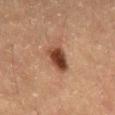follow-up = catalogued during a skin exam; not biopsied
lesion size = about 4 mm
subject = male, approximately 60 years of age
acquisition = total-body-photography crop, ~15 mm field of view
tile lighting = cross-polarized
image-analysis metrics = a mean CIELAB color near L≈39 a*≈20 b*≈28; a color-variation rating of about 7/10